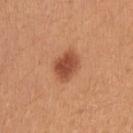workup — no biopsy performed (imaged during a skin exam)
subject — female, in their mid- to late 20s
tile lighting — white-light
image-analysis metrics — a footprint of about 7.5 mm², a shape eccentricity near 0.65, and a shape-asymmetry score of about 0.2 (0 = symmetric); a mean CIELAB color near L≈50 a*≈28 b*≈34, about 13 CIELAB-L* units darker than the surrounding skin, and a lesion-to-skin contrast of about 9 (normalized; higher = more distinct)
image source — 15 mm crop, total-body photography
anatomic site — the right upper arm
lesion size — about 3.5 mm